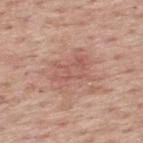Assessment: Captured during whole-body skin photography for melanoma surveillance; the lesion was not biopsied. Context: The total-body-photography lesion software estimated an area of roughly 15 mm², an eccentricity of roughly 0.75, and two-axis asymmetry of about 0.25. It also reported a lesion color around L≈58 a*≈23 b*≈26 in CIELAB, a lesion–skin lightness drop of about 7, and a normalized border contrast of about 5. A male subject, roughly 75 years of age. A 15 mm close-up extracted from a 3D total-body photography capture. The lesion is on the upper back.